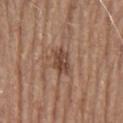biopsy_status: not biopsied; imaged during a skin examination
image:
  source: total-body photography crop
  field_of_view_mm: 15
patient:
  sex: female
  age_approx: 60
site: head or neck